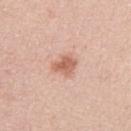Q: Is there a histopathology result?
A: no biopsy performed (imaged during a skin exam)
Q: How was the tile lit?
A: white-light illumination
Q: Lesion location?
A: the right upper arm
Q: What kind of image is this?
A: ~15 mm crop, total-body skin-cancer survey
Q: What did automated image analysis measure?
A: an average lesion color of about L≈63 a*≈23 b*≈30 (CIELAB), roughly 11 lightness units darker than nearby skin, and a lesion-to-skin contrast of about 7.5 (normalized; higher = more distinct)
Q: How large is the lesion?
A: ~3 mm (longest diameter)
Q: Patient demographics?
A: female, approximately 55 years of age